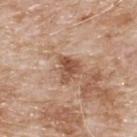No biopsy was performed on this lesion — it was imaged during a full skin examination and was not determined to be concerning. The patient is a male aged 78–82. A 15 mm close-up tile from a total-body photography series done for melanoma screening. The lesion is located on the upper back. The recorded lesion diameter is about 3 mm.